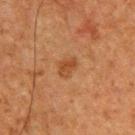Assessment:
This lesion was catalogued during total-body skin photography and was not selected for biopsy.
Clinical summary:
About 2.5 mm across. This image is a 15 mm lesion crop taken from a total-body photograph. A male patient aged around 50. Automated image analysis of the tile measured a lesion area of about 4 mm², a shape eccentricity near 0.75, and a shape-asymmetry score of about 0.3 (0 = symmetric). It also reported a border-irregularity rating of about 3/10 and a within-lesion color-variation index near 2.5/10. It also reported a classifier nevus-likeness of about 45/100 and a lesion-detection confidence of about 100/100. On the upper back.